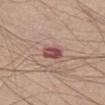biopsy status: imaged on a skin check; not biopsied | diameter: about 2.5 mm | illumination: white-light | image source: 15 mm crop, total-body photography | subject: male, aged approximately 65 | automated lesion analysis: a lesion area of about 5 mm², an outline eccentricity of about 0.65 (0 = round, 1 = elongated), and a symmetry-axis asymmetry near 0.2; a nevus-likeness score of about 5/100 and a detector confidence of about 100 out of 100 that the crop contains a lesion | site: the left thigh.